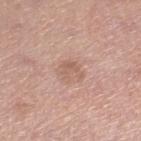lighting=white-light | acquisition=total-body-photography crop, ~15 mm field of view | lesion size=≈3 mm | anatomic site=the right lower leg | automated lesion analysis=a shape eccentricity near 0.65 and two-axis asymmetry of about 0.45; border irregularity of about 5.5 on a 0–10 scale, internal color variation of about 2 on a 0–10 scale, and peripheral color asymmetry of about 0.5; a lesion-detection confidence of about 100/100 | patient=male, aged 53 to 57.